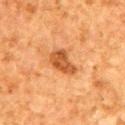Recorded during total-body skin imaging; not selected for excision or biopsy. A roughly 15 mm field-of-view crop from a total-body skin photograph. The patient is a male in their 80s. The lesion's longest dimension is about 4 mm. The tile uses cross-polarized illumination. The lesion is located on the upper back. Automated image analysis of the tile measured an area of roughly 6.5 mm² and a symmetry-axis asymmetry near 0.35. And it measured an average lesion color of about L≈43 a*≈24 b*≈37 (CIELAB) and a normalized border contrast of about 9. The analysis additionally found a border-irregularity index near 3/10, a color-variation rating of about 2/10, and peripheral color asymmetry of about 0.5. The analysis additionally found lesion-presence confidence of about 100/100.